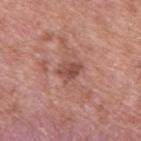The lesion was photographed on a routine skin check and not biopsied; there is no pathology result. Approximately 3 mm at its widest. Automated image analysis of the tile measured an area of roughly 4.5 mm², a shape eccentricity near 0.7, and two-axis asymmetry of about 0.25. It also reported a lesion color around L≈49 a*≈25 b*≈25 in CIELAB, a lesion–skin lightness drop of about 10, and a normalized border contrast of about 7. The software also gave a border-irregularity rating of about 2.5/10 and a within-lesion color-variation index near 3/10. From the upper back. A male patient aged around 55. This image is a 15 mm lesion crop taken from a total-body photograph.